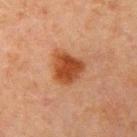Captured under cross-polarized illumination. About 4 mm across. A 15 mm close-up extracted from a 3D total-body photography capture. An algorithmic analysis of the crop reported an outline eccentricity of about 0.4 (0 = round, 1 = elongated) and a symmetry-axis asymmetry near 0.25. The software also gave an average lesion color of about L≈39 a*≈24 b*≈33 (CIELAB), a lesion–skin lightness drop of about 11, and a normalized border contrast of about 10. It also reported internal color variation of about 3.5 on a 0–10 scale and radial color variation of about 1. It also reported a classifier nevus-likeness of about 100/100 and a detector confidence of about 100 out of 100 that the crop contains a lesion. The lesion is on the arm. A male patient, roughly 70 years of age.